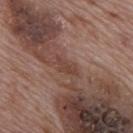follow-up: imaged on a skin check; not biopsied | subject: male, roughly 70 years of age | acquisition: total-body-photography crop, ~15 mm field of view | automated lesion analysis: an outline eccentricity of about 0.9 (0 = round, 1 = elongated) and a shape-asymmetry score of about 0.45 (0 = symmetric); a lesion–skin lightness drop of about 7 and a normalized border contrast of about 6; border irregularity of about 4.5 on a 0–10 scale, a within-lesion color-variation index near 1.5/10, and radial color variation of about 0.5; a classifier nevus-likeness of about 0/100 and a lesion-detection confidence of about 75/100 | body site: the mid back.